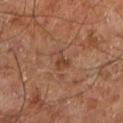<record>
<biopsy_status>not biopsied; imaged during a skin examination</biopsy_status>
<image>
  <source>total-body photography crop</source>
  <field_of_view_mm>15</field_of_view_mm>
</image>
<site>right leg</site>
<lighting>cross-polarized</lighting>
<patient>
  <sex>male</sex>
  <age_approx>60</age_approx>
</patient>
</record>